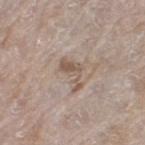follow-up: imaged on a skin check; not biopsied | acquisition: 15 mm crop, total-body photography | lesion size: ~4 mm (longest diameter) | subject: female, aged 73–77 | location: the left thigh | tile lighting: white-light.